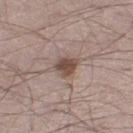<case>
<lighting>white-light</lighting>
<patient>
  <sex>male</sex>
  <age_approx>60</age_approx>
</patient>
<image>
  <source>total-body photography crop</source>
  <field_of_view_mm>15</field_of_view_mm>
</image>
<site>left thigh</site>
</case>